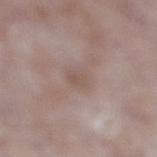A male patient about 75 years old.
The lesion is on the right lower leg.
A 15 mm close-up extracted from a 3D total-body photography capture.
Captured under white-light illumination.